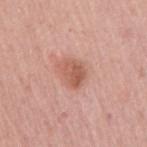Assessment:
The lesion was tiled from a total-body skin photograph and was not biopsied.
Clinical summary:
On the right upper arm. The recorded lesion diameter is about 3 mm. A male patient in their mid-60s. Automated image analysis of the tile measured a footprint of about 6.5 mm² and an outline eccentricity of about 0.65 (0 = round, 1 = elongated). The analysis additionally found an average lesion color of about L≈57 a*≈25 b*≈29 (CIELAB), roughly 10 lightness units darker than nearby skin, and a normalized lesion–skin contrast near 7. It also reported a classifier nevus-likeness of about 75/100 and a detector confidence of about 100 out of 100 that the crop contains a lesion. A 15 mm crop from a total-body photograph taken for skin-cancer surveillance. Captured under white-light illumination.